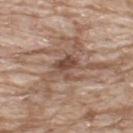Clinical impression: This lesion was catalogued during total-body skin photography and was not selected for biopsy. Context: The lesion is located on the upper back. Captured under white-light illumination. A 15 mm close-up extracted from a 3D total-body photography capture. The recorded lesion diameter is about 3 mm. The subject is a male in their mid-60s.